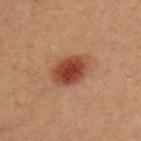Recorded during total-body skin imaging; not selected for excision or biopsy. The tile uses cross-polarized illumination. The lesion is on the upper back. A roughly 15 mm field-of-view crop from a total-body skin photograph. The patient is a female in their mid- to late 40s. About 4 mm across. An algorithmic analysis of the crop reported an outline eccentricity of about 0.75 (0 = round, 1 = elongated) and a shape-asymmetry score of about 0.1 (0 = symmetric). It also reported a border-irregularity rating of about 1/10 and a peripheral color-asymmetry measure near 1. It also reported lesion-presence confidence of about 100/100.